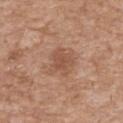– notes: catalogued during a skin exam; not biopsied
– illumination: white-light illumination
– site: the chest
– patient: male, aged 58–62
– lesion diameter: ≈3.5 mm
– imaging modality: 15 mm crop, total-body photography